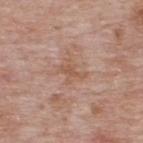Acquisition and patient details:
Automated image analysis of the tile measured a lesion area of about 4 mm², a shape eccentricity near 0.75, and a shape-asymmetry score of about 0.5 (0 = symmetric). And it measured a mean CIELAB color near L≈55 a*≈20 b*≈30, a lesion–skin lightness drop of about 7, and a normalized lesion–skin contrast near 6. And it measured a border-irregularity rating of about 7.5/10, internal color variation of about 0.5 on a 0–10 scale, and radial color variation of about 0. Located on the upper back. Measured at roughly 3 mm in maximum diameter. The tile uses white-light illumination. A male subject, aged around 65. A 15 mm close-up tile from a total-body photography series done for melanoma screening.